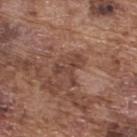Recorded during total-body skin imaging; not selected for excision or biopsy. Longest diameter approximately 3.5 mm. A 15 mm crop from a total-body photograph taken for skin-cancer surveillance. A male subject, roughly 75 years of age. This is a white-light tile. Automated tile analysis of the lesion measured an area of roughly 7 mm², an eccentricity of roughly 0.6, and a symmetry-axis asymmetry near 0.55. The software also gave an average lesion color of about L≈43 a*≈20 b*≈25 (CIELAB), about 8 CIELAB-L* units darker than the surrounding skin, and a normalized lesion–skin contrast near 6.5. The lesion is located on the back.